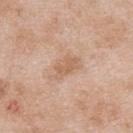follow-up: no biopsy performed (imaged during a skin exam); lesion size: about 3.5 mm; tile lighting: white-light; image source: 15 mm crop, total-body photography; site: the upper back; subject: male, about 25 years old.